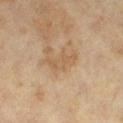The lesion was tiled from a total-body skin photograph and was not biopsied.
On the leg.
The lesion's longest dimension is about 4 mm.
A female patient, roughly 40 years of age.
Cropped from a total-body skin-imaging series; the visible field is about 15 mm.